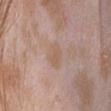No biopsy was performed on this lesion — it was imaged during a full skin examination and was not determined to be concerning.
Cropped from a total-body skin-imaging series; the visible field is about 15 mm.
The lesion is on the head or neck.
Imaged with white-light lighting.
The lesion's longest dimension is about 3 mm.
A female patient, approximately 25 years of age.